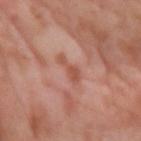Impression:
Recorded during total-body skin imaging; not selected for excision or biopsy.
Background:
The total-body-photography lesion software estimated an outline eccentricity of about 0.9 (0 = round, 1 = elongated). The software also gave a lesion color around L≈54 a*≈25 b*≈30 in CIELAB, about 8 CIELAB-L* units darker than the surrounding skin, and a normalized border contrast of about 6.5. The analysis additionally found a border-irregularity index near 6/10 and a peripheral color-asymmetry measure near 0. A lesion tile, about 15 mm wide, cut from a 3D total-body photograph. The lesion's longest dimension is about 3 mm. The lesion is located on the arm. Captured under cross-polarized illumination. A female patient aged approximately 55.